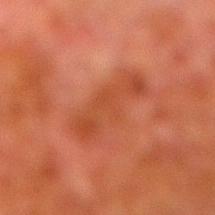{
  "biopsy_status": "not biopsied; imaged during a skin examination",
  "site": "right lower leg",
  "image": {
    "source": "total-body photography crop",
    "field_of_view_mm": 15
  },
  "patient": {
    "sex": "male",
    "age_approx": 80
  },
  "lighting": "cross-polarized",
  "lesion_size": {
    "long_diameter_mm_approx": 6.5
  },
  "automated_metrics": {
    "area_mm2_approx": 15.0,
    "eccentricity": 0.9,
    "shape_asymmetry": 0.25,
    "border_irregularity_0_10": 4.0,
    "color_variation_0_10": 2.5,
    "peripheral_color_asymmetry": 1.0
  }
}